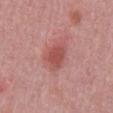<case>
  <biopsy_status>not biopsied; imaged during a skin examination</biopsy_status>
  <lighting>white-light</lighting>
  <patient>
    <sex>male</sex>
    <age_approx>50</age_approx>
  </patient>
  <image>
    <source>total-body photography crop</source>
    <field_of_view_mm>15</field_of_view_mm>
  </image>
  <site>abdomen</site>
  <lesion_size>
    <long_diameter_mm_approx>4.0</long_diameter_mm_approx>
  </lesion_size>
</case>